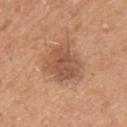Clinical impression:
Imaged during a routine full-body skin examination; the lesion was not biopsied and no histopathology is available.
Clinical summary:
A female patient, in their 40s. The lesion is located on the left upper arm. A close-up tile cropped from a whole-body skin photograph, about 15 mm across. Approximately 5 mm at its widest.